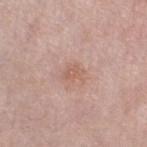Recorded during total-body skin imaging; not selected for excision or biopsy.